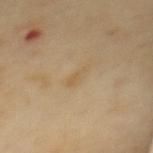Notes:
• workup: total-body-photography surveillance lesion; no biopsy
• imaging modality: 15 mm crop, total-body photography
• patient: female, about 60 years old
• body site: the back